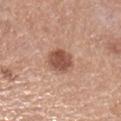notes: catalogued during a skin exam; not biopsied | acquisition: ~15 mm crop, total-body skin-cancer survey | patient: female, aged around 60 | lesion diameter: ~3 mm (longest diameter) | body site: the left forearm.